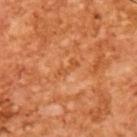Clinical impression:
Captured during whole-body skin photography for melanoma surveillance; the lesion was not biopsied.
Background:
Imaged with cross-polarized lighting. Cropped from a whole-body photographic skin survey; the tile spans about 15 mm. The lesion-visualizer software estimated an automated nevus-likeness rating near 0 out of 100 and a detector confidence of about 100 out of 100 that the crop contains a lesion. A male patient, in their mid- to late 60s. Longest diameter approximately 3 mm.